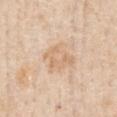notes — imaged on a skin check; not biopsied
illumination — white-light
location — the chest
subject — male, aged approximately 60
image source — ~15 mm tile from a whole-body skin photo